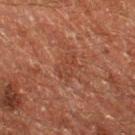Imaged during a routine full-body skin examination; the lesion was not biopsied and no histopathology is available. This image is a 15 mm lesion crop taken from a total-body photograph. Approximately 3.5 mm at its widest. The tile uses cross-polarized illumination. The total-body-photography lesion software estimated an area of roughly 5 mm² and a shape eccentricity near 0.8. The analysis additionally found internal color variation of about 1 on a 0–10 scale and peripheral color asymmetry of about 0.5. On the left thigh. A male patient, aged around 60.